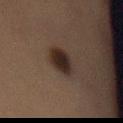Recorded during total-body skin imaging; not selected for excision or biopsy. From the abdomen. A lesion tile, about 15 mm wide, cut from a 3D total-body photograph. A female subject about 55 years old. Captured under cross-polarized illumination. An algorithmic analysis of the crop reported a footprint of about 7.5 mm², an eccentricity of roughly 0.85, and a shape-asymmetry score of about 0.15 (0 = symmetric). It also reported an average lesion color of about L≈20 a*≈11 b*≈16 (CIELAB), a lesion–skin lightness drop of about 10, and a normalized border contrast of about 12.5. About 4 mm across.